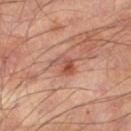| key | value |
|---|---|
| notes | imaged on a skin check; not biopsied |
| TBP lesion metrics | an average lesion color of about L≈48 a*≈24 b*≈28 (CIELAB) |
| illumination | cross-polarized illumination |
| diameter | ~3 mm (longest diameter) |
| site | the left thigh |
| patient | male, in their mid-50s |
| acquisition | total-body-photography crop, ~15 mm field of view |